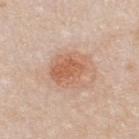Q: Was a biopsy performed?
A: catalogued during a skin exam; not biopsied
Q: How was this image acquired?
A: ~15 mm tile from a whole-body skin photo
Q: What is the anatomic site?
A: the upper back
Q: Who is the patient?
A: male, about 35 years old